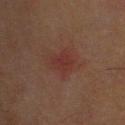{"lesion_size": {"long_diameter_mm_approx": 3.5}, "image": {"source": "total-body photography crop", "field_of_view_mm": 15}, "site": "head or neck", "automated_metrics": {"area_mm2_approx": 6.0, "eccentricity": 0.75, "shape_asymmetry": 0.25, "border_irregularity_0_10": 2.5, "nevus_likeness_0_100": 0}, "patient": {"sex": "male", "age_approx": 70}}